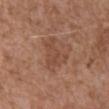{"biopsy_status": "not biopsied; imaged during a skin examination"}